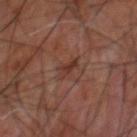Assessment:
Part of a total-body skin-imaging series; this lesion was reviewed on a skin check and was not flagged for biopsy.
Acquisition and patient details:
The patient is a male in their 60s. Cropped from a whole-body photographic skin survey; the tile spans about 15 mm. On the upper back. Approximately 3 mm at its widest. Captured under cross-polarized illumination.